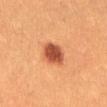<lesion>
<biopsy_status>not biopsied; imaged during a skin examination</biopsy_status>
</lesion>